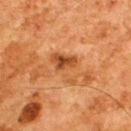Captured during whole-body skin photography for melanoma surveillance; the lesion was not biopsied. The patient is a male aged approximately 80. A 15 mm crop from a total-body photograph taken for skin-cancer surveillance. On the upper back.